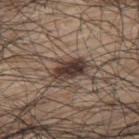Impression:
No biopsy was performed on this lesion — it was imaged during a full skin examination and was not determined to be concerning.
Acquisition and patient details:
A male patient, aged 68 to 72. Automated tile analysis of the lesion measured a classifier nevus-likeness of about 95/100. The lesion's longest dimension is about 4.5 mm. The lesion is on the upper back. The tile uses white-light illumination. A roughly 15 mm field-of-view crop from a total-body skin photograph.